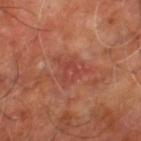Q: Is there a histopathology result?
A: imaged on a skin check; not biopsied
Q: Automated lesion metrics?
A: a footprint of about 9.5 mm², an outline eccentricity of about 0.55 (0 = round, 1 = elongated), and two-axis asymmetry of about 0.45; an average lesion color of about L≈45 a*≈29 b*≈29 (CIELAB) and a normalized border contrast of about 5; a border-irregularity rating of about 5.5/10, internal color variation of about 3.5 on a 0–10 scale, and radial color variation of about 1.5
Q: What are the patient's age and sex?
A: approximately 65 years of age
Q: Where on the body is the lesion?
A: the leg
Q: What is the imaging modality?
A: ~15 mm tile from a whole-body skin photo
Q: Lesion size?
A: about 4 mm